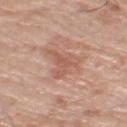  biopsy_status: not biopsied; imaged during a skin examination
  site: left thigh
  image:
    source: total-body photography crop
    field_of_view_mm: 15
  patient:
    sex: male
    age_approx: 80
  lesion_size:
    long_diameter_mm_approx: 5.0
  lighting: white-light